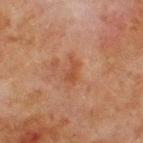Part of a total-body skin-imaging series; this lesion was reviewed on a skin check and was not flagged for biopsy. The lesion is on the chest. The tile uses cross-polarized illumination. This image is a 15 mm lesion crop taken from a total-body photograph. Measured at roughly 3 mm in maximum diameter. A male patient aged around 65.